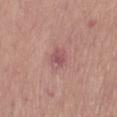Part of a total-body skin-imaging series; this lesion was reviewed on a skin check and was not flagged for biopsy.
A female patient, aged 68–72.
A 15 mm crop from a total-body photograph taken for skin-cancer surveillance.
Captured under white-light illumination.
Located on the right thigh.